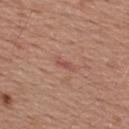Clinical impression: The lesion was photographed on a routine skin check and not biopsied; there is no pathology result. Context: A male subject aged around 55. A close-up tile cropped from a whole-body skin photograph, about 15 mm across. Captured under white-light illumination. Longest diameter approximately 3 mm. The lesion is located on the upper back.